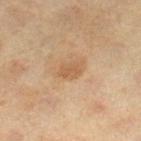<tbp_lesion>
<biopsy_status>not biopsied; imaged during a skin examination</biopsy_status>
<site>right lower leg</site>
<automated_metrics>
  <area_mm2_approx>4.0</area_mm2_approx>
  <eccentricity>0.75</eccentricity>
  <shape_asymmetry>0.35</shape_asymmetry>
  <nevus_likeness_0_100>30</nevus_likeness_0_100>
</automated_metrics>
<patient>
  <sex>female</sex>
  <age_approx>40</age_approx>
</patient>
<image>
  <source>total-body photography crop</source>
  <field_of_view_mm>15</field_of_view_mm>
</image>
<lesion_size>
  <long_diameter_mm_approx>2.5</long_diameter_mm_approx>
</lesion_size>
</tbp_lesion>